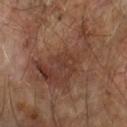Recorded during total-body skin imaging; not selected for excision or biopsy. An algorithmic analysis of the crop reported a lesion area of about 29 mm² and two-axis asymmetry of about 0.55. It also reported a border-irregularity rating of about 8/10, internal color variation of about 4.5 on a 0–10 scale, and a peripheral color-asymmetry measure near 1.5. A male subject approximately 65 years of age. Captured under cross-polarized illumination. This image is a 15 mm lesion crop taken from a total-body photograph. The lesion is located on the left forearm. Measured at roughly 10 mm in maximum diameter.